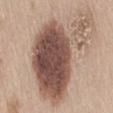Clinical impression:
The lesion was tiled from a total-body skin photograph and was not biopsied.
Context:
This is a white-light tile. A close-up tile cropped from a whole-body skin photograph, about 15 mm across. From the mid back. A female patient, aged around 50.